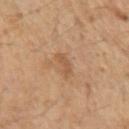Impression:
Imaged during a routine full-body skin examination; the lesion was not biopsied and no histopathology is available.
Acquisition and patient details:
An algorithmic analysis of the crop reported an area of roughly 3 mm², an outline eccentricity of about 0.85 (0 = round, 1 = elongated), and a shape-asymmetry score of about 0.35 (0 = symmetric). And it measured a lesion color around L≈44 a*≈17 b*≈29 in CIELAB, a lesion–skin lightness drop of about 6, and a lesion-to-skin contrast of about 5.5 (normalized; higher = more distinct). The analysis additionally found a color-variation rating of about 1/10 and a peripheral color-asymmetry measure near 0. The software also gave lesion-presence confidence of about 100/100. Located on the left forearm. The patient is a female about 55 years old. This is a cross-polarized tile. Approximately 2.5 mm at its widest. A lesion tile, about 15 mm wide, cut from a 3D total-body photograph.